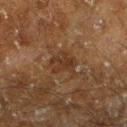{"biopsy_status": "not biopsied; imaged during a skin examination", "site": "left lower leg", "patient": {"sex": "male", "age_approx": 60}, "image": {"source": "total-body photography crop", "field_of_view_mm": 15}}